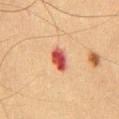{"biopsy_status": "not biopsied; imaged during a skin examination", "image": {"source": "total-body photography crop", "field_of_view_mm": 15}, "lighting": "cross-polarized", "site": "chest", "lesion_size": {"long_diameter_mm_approx": 3.5}, "patient": {"sex": "male", "age_approx": 65}}